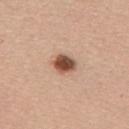{
  "biopsy_status": "not biopsied; imaged during a skin examination",
  "lighting": "white-light",
  "site": "mid back",
  "patient": {
    "sex": "female",
    "age_approx": 65
  },
  "lesion_size": {
    "long_diameter_mm_approx": 2.5
  },
  "image": {
    "source": "total-body photography crop",
    "field_of_view_mm": 15
  },
  "automated_metrics": {
    "cielab_L": 50,
    "cielab_a": 22,
    "cielab_b": 30,
    "vs_skin_darker_L": 19.0,
    "vs_skin_contrast_norm": 12.5,
    "peripheral_color_asymmetry": 2.0
  }
}